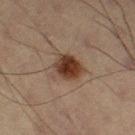{
  "biopsy_status": "not biopsied; imaged during a skin examination",
  "image": {
    "source": "total-body photography crop",
    "field_of_view_mm": 15
  },
  "lesion_size": {
    "long_diameter_mm_approx": 4.0
  },
  "patient": {
    "sex": "male",
    "age_approx": 55
  },
  "site": "right thigh"
}